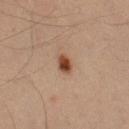Impression: No biopsy was performed on this lesion — it was imaged during a full skin examination and was not determined to be concerning. Image and clinical context: A male subject aged 33 to 37. The lesion is located on the left forearm. Automated tile analysis of the lesion measured about 13 CIELAB-L* units darker than the surrounding skin and a normalized lesion–skin contrast near 11. The analysis additionally found a within-lesion color-variation index near 5/10 and a peripheral color-asymmetry measure near 1.5. A region of skin cropped from a whole-body photographic capture, roughly 15 mm wide.